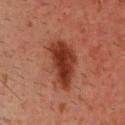No biopsy was performed on this lesion — it was imaged during a full skin examination and was not determined to be concerning. A male subject, about 50 years old. Automated tile analysis of the lesion measured a shape-asymmetry score of about 0.25 (0 = symmetric). Located on the head or neck. A close-up tile cropped from a whole-body skin photograph, about 15 mm across. Captured under cross-polarized illumination. The lesion's longest dimension is about 5.5 mm.